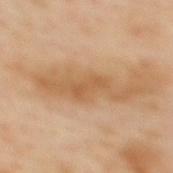Recorded during total-body skin imaging; not selected for excision or biopsy. The subject is a male in their mid-60s. On the mid back. Cropped from a total-body skin-imaging series; the visible field is about 15 mm.